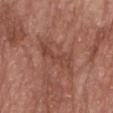Captured during whole-body skin photography for melanoma surveillance; the lesion was not biopsied. Imaged with white-light lighting. A male subject aged 63–67. A region of skin cropped from a whole-body photographic capture, roughly 15 mm wide. Measured at roughly 6 mm in maximum diameter. From the head or neck.